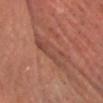Q: Was this lesion biopsied?
A: total-body-photography surveillance lesion; no biopsy
Q: What did automated image analysis measure?
A: an area of roughly 11 mm² and a shape-asymmetry score of about 0.25 (0 = symmetric); an automated nevus-likeness rating near 0 out of 100
Q: Who is the patient?
A: male, aged approximately 65
Q: Lesion location?
A: the head or neck
Q: What is the imaging modality?
A: ~15 mm tile from a whole-body skin photo
Q: Illumination type?
A: cross-polarized illumination